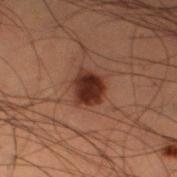  biopsy_status: not biopsied; imaged during a skin examination
  site: left thigh
  lesion_size:
    long_diameter_mm_approx: 3.5
  image:
    source: total-body photography crop
    field_of_view_mm: 15
  lighting: cross-polarized
  patient:
    sex: male
    age_approx: 50